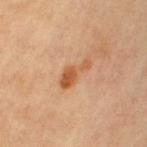Cropped from a total-body skin-imaging series; the visible field is about 15 mm.
A female patient, in their mid-60s.
Located on the left upper arm.